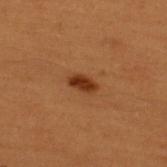biopsy status: no biopsy performed (imaged during a skin exam)
site: the upper back
acquisition: ~15 mm crop, total-body skin-cancer survey
patient: male, aged approximately 50